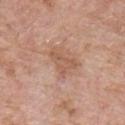- workup: catalogued during a skin exam; not biopsied
- lesion size: about 3.5 mm
- patient: male, about 60 years old
- imaging modality: 15 mm crop, total-body photography
- tile lighting: white-light illumination
- automated metrics: an area of roughly 6.5 mm² and an eccentricity of roughly 0.75
- body site: the front of the torso